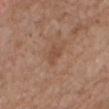The lesion was tiled from a total-body skin photograph and was not biopsied. This is a white-light tile. A 15 mm close-up extracted from a 3D total-body photography capture. Located on the head or neck. The recorded lesion diameter is about 2.5 mm. A male patient aged 68–72. The lesion-visualizer software estimated an area of roughly 3.5 mm² and two-axis asymmetry of about 0.35. The analysis additionally found about 7 CIELAB-L* units darker than the surrounding skin and a lesion-to-skin contrast of about 5.5 (normalized; higher = more distinct).